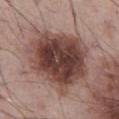{
  "site": "abdomen",
  "patient": {
    "sex": "male",
    "age_approx": 55
  },
  "image": {
    "source": "total-body photography crop",
    "field_of_view_mm": 15
  }
}